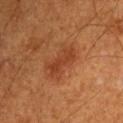{"lesion_size": {"long_diameter_mm_approx": 4.5}, "automated_metrics": {"border_irregularity_0_10": 3.5, "color_variation_0_10": 2.5, "peripheral_color_asymmetry": 1.0, "nevus_likeness_0_100": 45}, "image": {"source": "total-body photography crop", "field_of_view_mm": 15}, "site": "left upper arm", "lighting": "cross-polarized", "patient": {"sex": "male", "age_approx": 60}}